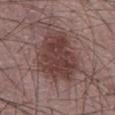Recorded during total-body skin imaging; not selected for excision or biopsy. A 15 mm close-up tile from a total-body photography series done for melanoma screening. The lesion is located on the abdomen. Longest diameter approximately 7.5 mm. A male subject, about 60 years old.